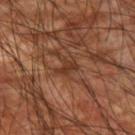Impression:
The lesion was photographed on a routine skin check and not biopsied; there is no pathology result.
Acquisition and patient details:
Approximately 3 mm at its widest. From the right upper arm. A male patient approximately 60 years of age. Imaged with cross-polarized lighting. A region of skin cropped from a whole-body photographic capture, roughly 15 mm wide.